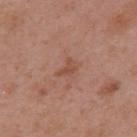follow-up: imaged on a skin check; not biopsied
lesion diameter: about 2.5 mm
anatomic site: the upper back
tile lighting: white-light
image source: total-body-photography crop, ~15 mm field of view
patient: female, aged approximately 40